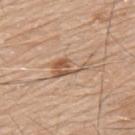Impression:
Imaged during a routine full-body skin examination; the lesion was not biopsied and no histopathology is available.
Background:
Longest diameter approximately 4.5 mm. The lesion is on the mid back. A male subject aged 78–82. Captured under white-light illumination. A roughly 15 mm field-of-view crop from a total-body skin photograph.